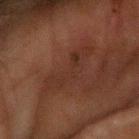• workup — total-body-photography surveillance lesion; no biopsy
• size — about 6 mm
• illumination — cross-polarized illumination
• subject — male, aged approximately 65
• anatomic site — the left forearm
• imaging modality — ~15 mm crop, total-body skin-cancer survey
• TBP lesion metrics — a border-irregularity index near 7.5/10 and a peripheral color-asymmetry measure near 1; a detector confidence of about 100 out of 100 that the crop contains a lesion